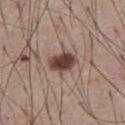A male subject in their mid-50s. A close-up tile cropped from a whole-body skin photograph, about 15 mm across. The tile uses white-light illumination. Automated tile analysis of the lesion measured an area of roughly 7.5 mm², a shape eccentricity near 0.7, and a symmetry-axis asymmetry near 0.25. And it measured roughly 16 lightness units darker than nearby skin and a normalized border contrast of about 12. And it measured an automated nevus-likeness rating near 100 out of 100. Measured at roughly 3.5 mm in maximum diameter. On the abdomen.